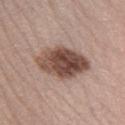- notes: total-body-photography surveillance lesion; no biopsy
- image source: ~15 mm tile from a whole-body skin photo
- anatomic site: the leg
- lighting: white-light illumination
- automated metrics: a color-variation rating of about 7.5/10 and peripheral color asymmetry of about 3
- lesion size: about 6.5 mm
- patient: female, aged around 40